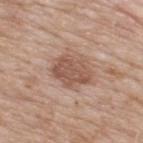notes: no biopsy performed (imaged during a skin exam) | automated metrics: a footprint of about 11 mm² and a shape-asymmetry score of about 0.25 (0 = symmetric); an automated nevus-likeness rating near 30 out of 100 and a lesion-detection confidence of about 100/100 | lesion size: ~4.5 mm (longest diameter) | anatomic site: the upper back | patient: male, about 65 years old | imaging modality: total-body-photography crop, ~15 mm field of view | illumination: white-light illumination.